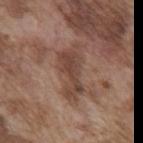Findings:
• workup: total-body-photography surveillance lesion; no biopsy
• site: the chest
• image source: 15 mm crop, total-body photography
• tile lighting: white-light
• subject: male, in their mid- to late 70s
• lesion diameter: ~6 mm (longest diameter)